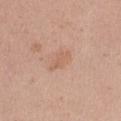notes = no biopsy performed (imaged during a skin exam) | lighting = white-light illumination | location = the right thigh | patient = female, roughly 30 years of age | image source = total-body-photography crop, ~15 mm field of view.